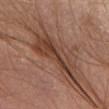The lesion was tiled from a total-body skin photograph and was not biopsied.